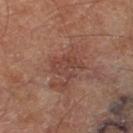Impression:
Captured during whole-body skin photography for melanoma surveillance; the lesion was not biopsied.
Acquisition and patient details:
A 15 mm close-up extracted from a 3D total-body photography capture. Measured at roughly 3.5 mm in maximum diameter. The lesion is located on the right thigh. The subject is a male approximately 70 years of age.